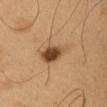The lesion was tiled from a total-body skin photograph and was not biopsied. A lesion tile, about 15 mm wide, cut from a 3D total-body photograph. The subject is a male aged 48 to 52. Located on the right upper arm.